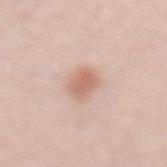Clinical summary:
The subject is a male in their 50s. Cropped from a whole-body photographic skin survey; the tile spans about 15 mm. On the lower back.